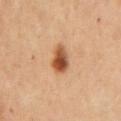Imaged during a routine full-body skin examination; the lesion was not biopsied and no histopathology is available. Cropped from a whole-body photographic skin survey; the tile spans about 15 mm. Approximately 3.5 mm at its widest. The subject is a female roughly 45 years of age. On the right upper arm.